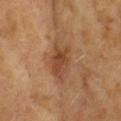Part of a total-body skin-imaging series; this lesion was reviewed on a skin check and was not flagged for biopsy. The lesion is located on the head or neck. Longest diameter approximately 5 mm. A 15 mm close-up tile from a total-body photography series done for melanoma screening. The subject is a female in their 60s. The tile uses cross-polarized illumination.